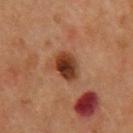| key | value |
|---|---|
| workup | imaged on a skin check; not biopsied |
| subject | male, about 60 years old |
| body site | the upper back |
| lesion size | ~4 mm (longest diameter) |
| imaging modality | total-body-photography crop, ~15 mm field of view |
| illumination | cross-polarized illumination |